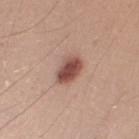{
  "biopsy_status": "not biopsied; imaged during a skin examination",
  "automated_metrics": {
    "cielab_L": 50,
    "cielab_a": 22,
    "cielab_b": 25,
    "vs_skin_darker_L": 15.0,
    "border_irregularity_0_10": 1.5,
    "color_variation_0_10": 4.0,
    "peripheral_color_asymmetry": 1.5,
    "nevus_likeness_0_100": 95,
    "lesion_detection_confidence_0_100": 100
  },
  "image": {
    "source": "total-body photography crop",
    "field_of_view_mm": 15
  },
  "patient": {
    "sex": "male",
    "age_approx": 25
  },
  "site": "left upper arm",
  "lesion_size": {
    "long_diameter_mm_approx": 3.5
  }
}